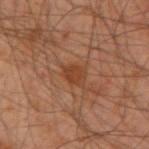Case summary:
* notes — no biopsy performed (imaged during a skin exam)